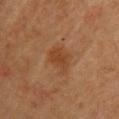biopsy_status: not biopsied; imaged during a skin examination
patient:
  sex: female
  age_approx: 60
lesion_size:
  long_diameter_mm_approx: 3.5
image:
  source: total-body photography crop
  field_of_view_mm: 15
lighting: cross-polarized
automated_metrics:
  area_mm2_approx: 8.0
  eccentricity: 0.55
  shape_asymmetry: 0.3
  cielab_L: 38
  cielab_a: 20
  cielab_b: 31
  lesion_detection_confidence_0_100: 100
site: upper back